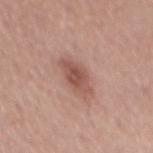Assessment:
No biopsy was performed on this lesion — it was imaged during a full skin examination and was not determined to be concerning.
Acquisition and patient details:
Imaged with white-light lighting. Located on the mid back. A male patient roughly 55 years of age. Cropped from a total-body skin-imaging series; the visible field is about 15 mm. The lesion's longest dimension is about 4.5 mm.